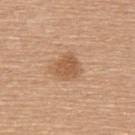Clinical impression: This lesion was catalogued during total-body skin photography and was not selected for biopsy. Image and clinical context: Captured under white-light illumination. This image is a 15 mm lesion crop taken from a total-body photograph. The lesion is located on the upper back. Approximately 3 mm at its widest. A female patient, approximately 60 years of age. Automated tile analysis of the lesion measured an average lesion color of about L≈57 a*≈20 b*≈35 (CIELAB), roughly 10 lightness units darker than nearby skin, and a normalized lesion–skin contrast near 7. The analysis additionally found border irregularity of about 2 on a 0–10 scale and radial color variation of about 0.5.